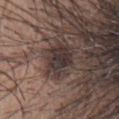The lesion was tiled from a total-body skin photograph and was not biopsied. Measured at roughly 4.5 mm in maximum diameter. A female patient, aged approximately 40. Captured under white-light illumination. Automated image analysis of the tile measured lesion-presence confidence of about 30/100. Cropped from a total-body skin-imaging series; the visible field is about 15 mm. The lesion is on the abdomen.